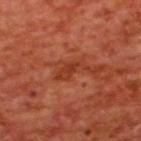Part of a total-body skin-imaging series; this lesion was reviewed on a skin check and was not flagged for biopsy. A lesion tile, about 15 mm wide, cut from a 3D total-body photograph. The tile uses cross-polarized illumination. Measured at roughly 4 mm in maximum diameter. Automated tile analysis of the lesion measured a lesion area of about 4.5 mm² and a shape eccentricity near 0.9. The analysis additionally found a border-irregularity rating of about 5/10 and radial color variation of about 0.5. And it measured a nevus-likeness score of about 0/100 and lesion-presence confidence of about 100/100. Located on the back. The subject is a male about 65 years old.